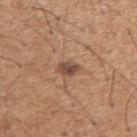The lesion was photographed on a routine skin check and not biopsied; there is no pathology result. The lesion is located on the right upper arm. The patient is a male roughly 65 years of age. A roughly 15 mm field-of-view crop from a total-body skin photograph.